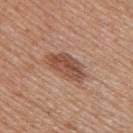Impression:
Part of a total-body skin-imaging series; this lesion was reviewed on a skin check and was not flagged for biopsy.
Context:
The tile uses white-light illumination. Longest diameter approximately 5 mm. The patient is a female aged 48–52. Automated image analysis of the tile measured an average lesion color of about L≈50 a*≈22 b*≈29 (CIELAB), a lesion–skin lightness drop of about 11, and a lesion-to-skin contrast of about 8 (normalized; higher = more distinct). It also reported border irregularity of about 3 on a 0–10 scale and radial color variation of about 1.5. On the upper back. A region of skin cropped from a whole-body photographic capture, roughly 15 mm wide.